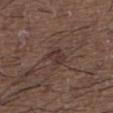Impression:
Recorded during total-body skin imaging; not selected for excision or biopsy.
Clinical summary:
A 15 mm crop from a total-body photograph taken for skin-cancer surveillance. Located on the back. A male subject, aged approximately 50.